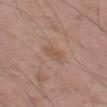biopsy status: no biopsy performed (imaged during a skin exam) | size: ~2.5 mm (longest diameter) | acquisition: total-body-photography crop, ~15 mm field of view | image-analysis metrics: a shape-asymmetry score of about 0.35 (0 = symmetric); a lesion–skin lightness drop of about 6 and a lesion-to-skin contrast of about 5 (normalized; higher = more distinct); an automated nevus-likeness rating near 0 out of 100 and a lesion-detection confidence of about 100/100 | anatomic site: the left thigh | subject: male, approximately 50 years of age.